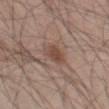| feature | finding |
|---|---|
| biopsy status | total-body-photography surveillance lesion; no biopsy |
| lighting | white-light |
| location | the leg |
| lesion diameter | about 3 mm |
| automated metrics | a mean CIELAB color near L≈47 a*≈18 b*≈25 and about 9 CIELAB-L* units darker than the surrounding skin; a classifier nevus-likeness of about 55/100 and a detector confidence of about 100 out of 100 that the crop contains a lesion |
| subject | male, approximately 45 years of age |
| image | ~15 mm tile from a whole-body skin photo |